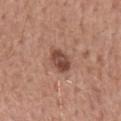– size — ~3 mm (longest diameter)
– image source — ~15 mm tile from a whole-body skin photo
– anatomic site — the back
– tile lighting — white-light
– automated lesion analysis — an area of roughly 6.5 mm², a shape eccentricity near 0.65, and a shape-asymmetry score of about 0.2 (0 = symmetric); roughly 12 lightness units darker than nearby skin and a lesion-to-skin contrast of about 9 (normalized; higher = more distinct); a color-variation rating of about 4.5/10 and a peripheral color-asymmetry measure near 1.5; a nevus-likeness score of about 60/100
– subject — male, aged approximately 55